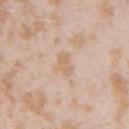No biopsy was performed on this lesion — it was imaged during a full skin examination and was not determined to be concerning.
Approximately 2.5 mm at its widest.
A close-up tile cropped from a whole-body skin photograph, about 15 mm across.
The lesion is located on the right upper arm.
The patient is a female in their mid-20s.
Imaged with white-light lighting.